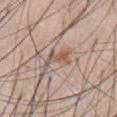Assessment: Imaged during a routine full-body skin examination; the lesion was not biopsied and no histopathology is available. Clinical summary: A 15 mm close-up extracted from a 3D total-body photography capture. A male patient, approximately 60 years of age. This is a white-light tile. From the abdomen. The lesion-visualizer software estimated a footprint of about 4.5 mm², a shape eccentricity near 0.85, and two-axis asymmetry of about 0.55. The analysis additionally found roughly 9 lightness units darker than nearby skin and a normalized lesion–skin contrast near 7. The analysis additionally found a border-irregularity index near 6.5/10, internal color variation of about 2.5 on a 0–10 scale, and peripheral color asymmetry of about 0.5. And it measured lesion-presence confidence of about 80/100.